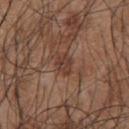Assessment:
Captured during whole-body skin photography for melanoma surveillance; the lesion was not biopsied.
Background:
About 2.5 mm across. A close-up tile cropped from a whole-body skin photograph, about 15 mm across. The subject is a male about 55 years old. This is a white-light tile. The lesion is on the chest.